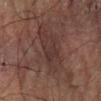- workup · no biopsy performed (imaged during a skin exam)
- automated lesion analysis · an area of roughly 13 mm² and a symmetry-axis asymmetry near 0.3; a lesion–skin lightness drop of about 5 and a normalized lesion–skin contrast near 6; a border-irregularity rating of about 4.5/10 and a color-variation rating of about 2.5/10; a lesion-detection confidence of about 75/100
- image source · ~15 mm crop, total-body skin-cancer survey
- illumination · cross-polarized
- subject · male, aged around 55
- body site · the leg
- size · ≈6.5 mm